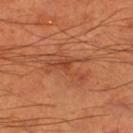No biopsy was performed on this lesion — it was imaged during a full skin examination and was not determined to be concerning. From the left thigh. Automated image analysis of the tile measured a border-irregularity rating of about 9/10, internal color variation of about 4 on a 0–10 scale, and peripheral color asymmetry of about 1. The lesion's longest dimension is about 5 mm. This image is a 15 mm lesion crop taken from a total-body photograph. A male patient, approximately 55 years of age.